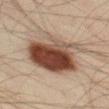follow-up: imaged on a skin check; not biopsied | image: ~15 mm crop, total-body skin-cancer survey | site: the left thigh | illumination: cross-polarized illumination | size: ~7.5 mm (longest diameter) | patient: male, in their 40s.